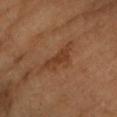Imaged during a routine full-body skin examination; the lesion was not biopsied and no histopathology is available. Imaged with cross-polarized lighting. About 5.5 mm across. A 15 mm crop from a total-body photograph taken for skin-cancer surveillance. On the left forearm. A female subject, roughly 55 years of age.